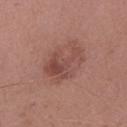Q: Is there a histopathology result?
A: total-body-photography surveillance lesion; no biopsy
Q: What did automated image analysis measure?
A: a footprint of about 14 mm² and a shape eccentricity near 0.7; border irregularity of about 5.5 on a 0–10 scale and internal color variation of about 6 on a 0–10 scale; a detector confidence of about 100 out of 100 that the crop contains a lesion
Q: How was this image acquired?
A: ~15 mm crop, total-body skin-cancer survey
Q: What are the patient's age and sex?
A: female, aged 38 to 42
Q: Lesion location?
A: the right forearm